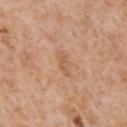follow-up: no biopsy performed (imaged during a skin exam)
image: ~15 mm crop, total-body skin-cancer survey
tile lighting: white-light
patient: male, aged around 65
anatomic site: the front of the torso
TBP lesion metrics: a border-irregularity rating of about 3.5/10, a color-variation rating of about 1/10, and radial color variation of about 0.5
diameter: about 3.5 mm